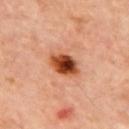Part of a total-body skin-imaging series; this lesion was reviewed on a skin check and was not flagged for biopsy.
The subject is a male roughly 60 years of age.
Automated tile analysis of the lesion measured border irregularity of about 1.5 on a 0–10 scale, internal color variation of about 10 on a 0–10 scale, and peripheral color asymmetry of about 3.5.
The lesion is located on the upper back.
Longest diameter approximately 4 mm.
Captured under cross-polarized illumination.
A lesion tile, about 15 mm wide, cut from a 3D total-body photograph.